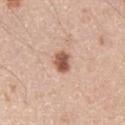follow-up: total-body-photography surveillance lesion; no biopsy
location: the left upper arm
patient: male, in their mid- to late 40s
image: total-body-photography crop, ~15 mm field of view
diameter: ~2.5 mm (longest diameter)
tile lighting: white-light illumination